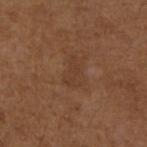Clinical impression:
Part of a total-body skin-imaging series; this lesion was reviewed on a skin check and was not flagged for biopsy.
Context:
The lesion is located on the left upper arm. Captured under white-light illumination. A male patient approximately 75 years of age. Cropped from a total-body skin-imaging series; the visible field is about 15 mm.